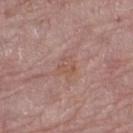Clinical impression:
This lesion was catalogued during total-body skin photography and was not selected for biopsy.
Clinical summary:
The lesion's longest dimension is about 2.5 mm. The subject is a female roughly 65 years of age. An algorithmic analysis of the crop reported a border-irregularity index near 3/10, internal color variation of about 3 on a 0–10 scale, and radial color variation of about 1. And it measured an automated nevus-likeness rating near 0 out of 100. From the left thigh. A 15 mm crop from a total-body photograph taken for skin-cancer surveillance.